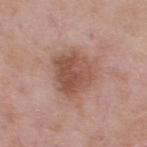image-analysis metrics: an eccentricity of roughly 0.45 and a shape-asymmetry score of about 0.25 (0 = symmetric); border irregularity of about 2.5 on a 0–10 scale, a color-variation rating of about 4/10, and radial color variation of about 1.5; a classifier nevus-likeness of about 20/100 and a detector confidence of about 100 out of 100 that the crop contains a lesion
size: about 5 mm
subject: male, in their mid-50s
site: the upper back
acquisition: ~15 mm tile from a whole-body skin photo
illumination: white-light